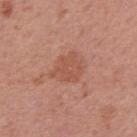Recorded during total-body skin imaging; not selected for excision or biopsy. A female patient aged around 50. Imaged with white-light lighting. On the right upper arm. A 15 mm crop from a total-body photograph taken for skin-cancer surveillance.